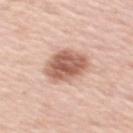No biopsy was performed on this lesion — it was imaged during a full skin examination and was not determined to be concerning. Automated image analysis of the tile measured a lesion–skin lightness drop of about 15. The software also gave border irregularity of about 2 on a 0–10 scale and radial color variation of about 2. Cropped from a whole-body photographic skin survey; the tile spans about 15 mm. Located on the right upper arm. Approximately 5 mm at its widest. The subject is a female roughly 50 years of age.